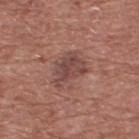notes: catalogued during a skin exam; not biopsied | image: ~15 mm crop, total-body skin-cancer survey | TBP lesion metrics: a lesion area of about 9.5 mm² and an outline eccentricity of about 0.75 (0 = round, 1 = elongated); a lesion color around L≈45 a*≈21 b*≈22 in CIELAB; internal color variation of about 4 on a 0–10 scale and radial color variation of about 1.5; an automated nevus-likeness rating near 10 out of 100 and lesion-presence confidence of about 100/100 | body site: the upper back | tile lighting: white-light | patient: male, aged approximately 75.